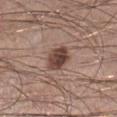Clinical impression:
Recorded during total-body skin imaging; not selected for excision or biopsy.